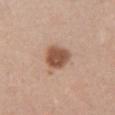Captured during whole-body skin photography for melanoma surveillance; the lesion was not biopsied.
The patient is a female aged 28 to 32.
Captured under white-light illumination.
A roughly 15 mm field-of-view crop from a total-body skin photograph.
From the chest.